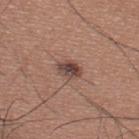Impression:
The lesion was tiled from a total-body skin photograph and was not biopsied.
Acquisition and patient details:
A male subject, aged 33–37. Cropped from a total-body skin-imaging series; the visible field is about 15 mm. Longest diameter approximately 2.5 mm. Located on the upper back.